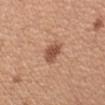Clinical impression:
Imaged during a routine full-body skin examination; the lesion was not biopsied and no histopathology is available.
Image and clinical context:
A 15 mm crop from a total-body photograph taken for skin-cancer surveillance. Measured at roughly 3 mm in maximum diameter. The lesion is located on the arm. A female subject, aged 33 to 37. Automated image analysis of the tile measured a lesion color around L≈53 a*≈22 b*≈31 in CIELAB and a normalized border contrast of about 8. It also reported a color-variation rating of about 2.5/10 and radial color variation of about 0.5. It also reported a classifier nevus-likeness of about 90/100. This is a white-light tile.